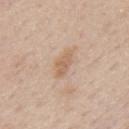Clinical impression:
Part of a total-body skin-imaging series; this lesion was reviewed on a skin check and was not flagged for biopsy.
Background:
A 15 mm close-up extracted from a 3D total-body photography capture. Approximately 3.5 mm at its widest. A male subject, approximately 60 years of age. Captured under white-light illumination. The lesion is on the mid back.